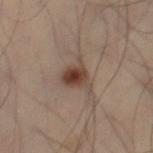* notes: catalogued during a skin exam; not biopsied
* illumination: cross-polarized illumination
* location: the lower back
* image: total-body-photography crop, ~15 mm field of view
* subject: male, about 50 years old